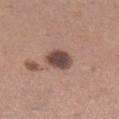Notes:
– follow-up · catalogued during a skin exam; not biopsied
– lesion diameter · ~3.5 mm (longest diameter)
– body site · the right lower leg
– patient · female, in their 30s
– tile lighting · white-light illumination
– acquisition · ~15 mm crop, total-body skin-cancer survey